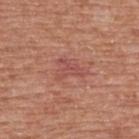Q: Is there a histopathology result?
A: no biopsy performed (imaged during a skin exam)
Q: What are the patient's age and sex?
A: male, aged approximately 65
Q: What is the imaging modality?
A: 15 mm crop, total-body photography
Q: What is the lesion's diameter?
A: ~4 mm (longest diameter)
Q: What is the anatomic site?
A: the upper back
Q: Illumination type?
A: white-light illumination